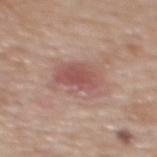Captured during whole-body skin photography for melanoma surveillance; the lesion was not biopsied. A close-up tile cropped from a whole-body skin photograph, about 15 mm across. A female subject, about 60 years old. Automated tile analysis of the lesion measured an area of roughly 12 mm², an eccentricity of roughly 0.8, and a shape-asymmetry score of about 0.25 (0 = symmetric). It also reported an average lesion color of about L≈53 a*≈23 b*≈24 (CIELAB) and roughly 10 lightness units darker than nearby skin. The software also gave a nevus-likeness score of about 80/100 and a lesion-detection confidence of about 100/100. On the upper back.